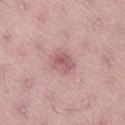biopsy status — no biopsy performed (imaged during a skin exam) | acquisition — ~15 mm crop, total-body skin-cancer survey | automated metrics — an area of roughly 6.5 mm²; a border-irregularity index near 2.5/10, internal color variation of about 4 on a 0–10 scale, and peripheral color asymmetry of about 1.5; a lesion-detection confidence of about 100/100 | site — the lower back | patient — female, aged around 40 | lighting — white-light illumination.